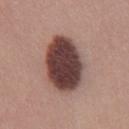From the front of the torso.
The lesion's longest dimension is about 7 mm.
A male patient, aged 33 to 37.
Imaged with white-light lighting.
A region of skin cropped from a whole-body photographic capture, roughly 15 mm wide.